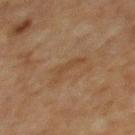The lesion was tiled from a total-body skin photograph and was not biopsied. A female patient in their 60s. Located on the upper back. A 15 mm crop from a total-body photograph taken for skin-cancer surveillance.